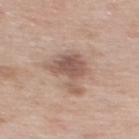The lesion was photographed on a routine skin check and not biopsied; there is no pathology result.
Cropped from a whole-body photographic skin survey; the tile spans about 15 mm.
Automated image analysis of the tile measured an area of roughly 15 mm², an outline eccentricity of about 0.7 (0 = round, 1 = elongated), and a shape-asymmetry score of about 0.45 (0 = symmetric). The software also gave an average lesion color of about L≈56 a*≈18 b*≈25 (CIELAB).
The patient is a female roughly 40 years of age.
Approximately 5 mm at its widest.
This is a white-light tile.
The lesion is located on the upper back.